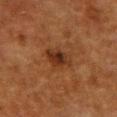From the front of the torso.
Approximately 3.5 mm at its widest.
A 15 mm crop from a total-body photograph taken for skin-cancer surveillance.
Automated image analysis of the tile measured an area of roughly 6.5 mm² and two-axis asymmetry of about 0.3. The software also gave a border-irregularity rating of about 3.5/10 and a color-variation rating of about 5/10.
Captured under cross-polarized illumination.
The subject is a female approximately 50 years of age.